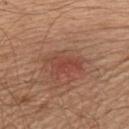biopsy status: catalogued during a skin exam; not biopsied | image source: total-body-photography crop, ~15 mm field of view | lesion diameter: ≈4 mm | subject: male, aged around 65 | site: the left upper arm | tile lighting: white-light illumination.